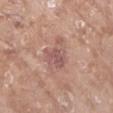- workup — no biopsy performed (imaged during a skin exam)
- body site — the arm
- subject — female, about 75 years old
- image source — ~15 mm tile from a whole-body skin photo